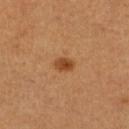workup: total-body-photography surveillance lesion; no biopsy
patient: female, approximately 50 years of age
image: 15 mm crop, total-body photography
tile lighting: cross-polarized illumination
size: about 2.5 mm
location: the right lower leg